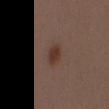Part of a total-body skin-imaging series; this lesion was reviewed on a skin check and was not flagged for biopsy. A male subject aged approximately 40. The lesion is located on the mid back. A region of skin cropped from a whole-body photographic capture, roughly 15 mm wide. Captured under white-light illumination.